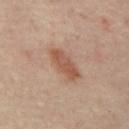Q: Is there a histopathology result?
A: total-body-photography surveillance lesion; no biopsy
Q: What is the imaging modality?
A: total-body-photography crop, ~15 mm field of view
Q: What is the anatomic site?
A: the mid back
Q: What are the patient's age and sex?
A: aged around 55